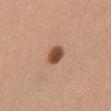Part of a total-body skin-imaging series; this lesion was reviewed on a skin check and was not flagged for biopsy. A roughly 15 mm field-of-view crop from a total-body skin photograph. Located on the abdomen. About 2.5 mm across. An algorithmic analysis of the crop reported an area of roughly 5 mm² and a shape eccentricity near 0.6. The software also gave a classifier nevus-likeness of about 100/100 and a detector confidence of about 100 out of 100 that the crop contains a lesion. A female patient, approximately 55 years of age.